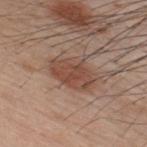| field | value |
|---|---|
| follow-up | total-body-photography surveillance lesion; no biopsy |
| automated lesion analysis | a footprint of about 12 mm², an eccentricity of roughly 0.8, and a symmetry-axis asymmetry near 0.2; a lesion–skin lightness drop of about 10 and a normalized border contrast of about 7.5; lesion-presence confidence of about 100/100 |
| patient | male, roughly 35 years of age |
| diameter | ≈5 mm |
| image source | ~15 mm tile from a whole-body skin photo |
| location | the back |
| tile lighting | white-light |